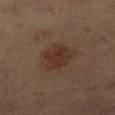Impression:
Recorded during total-body skin imaging; not selected for excision or biopsy.
Context:
Cropped from a total-body skin-imaging series; the visible field is about 15 mm. Approximately 4.5 mm at its widest. Located on the leg. The total-body-photography lesion software estimated roughly 7 lightness units darker than nearby skin. The analysis additionally found a border-irregularity rating of about 2/10, a color-variation rating of about 2.5/10, and radial color variation of about 1. The software also gave a nevus-likeness score of about 95/100 and a detector confidence of about 100 out of 100 that the crop contains a lesion. A female patient, aged 68–72. Imaged with cross-polarized lighting.